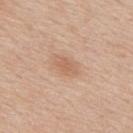Imaged during a routine full-body skin examination; the lesion was not biopsied and no histopathology is available.
The tile uses white-light illumination.
On the back.
A close-up tile cropped from a whole-body skin photograph, about 15 mm across.
The lesion's longest dimension is about 2.5 mm.
A male subject in their 60s.